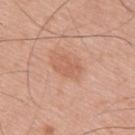No biopsy was performed on this lesion — it was imaged during a full skin examination and was not determined to be concerning.
A 15 mm close-up tile from a total-body photography series done for melanoma screening.
The tile uses white-light illumination.
From the upper back.
The total-body-photography lesion software estimated a mean CIELAB color near L≈60 a*≈24 b*≈32, about 7 CIELAB-L* units darker than the surrounding skin, and a normalized border contrast of about 5. The analysis additionally found an automated nevus-likeness rating near 5 out of 100 and lesion-presence confidence of about 100/100.
A male subject aged 53 to 57.
Approximately 3 mm at its widest.